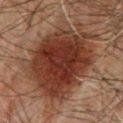Impression:
Imaged during a routine full-body skin examination; the lesion was not biopsied and no histopathology is available.
Context:
A 15 mm close-up extracted from a 3D total-body photography capture. Automated tile analysis of the lesion measured a shape-asymmetry score of about 0.15 (0 = symmetric). Located on the chest. Imaged with cross-polarized lighting. A male subject, approximately 60 years of age. The lesion's longest dimension is about 10 mm.